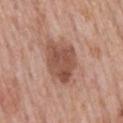The lesion was photographed on a routine skin check and not biopsied; there is no pathology result. From the mid back. Longest diameter approximately 5 mm. A male patient, aged around 75. A roughly 15 mm field-of-view crop from a total-body skin photograph.